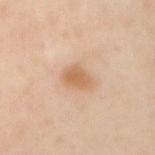Impression: Recorded during total-body skin imaging; not selected for excision or biopsy. Background: Imaged with cross-polarized lighting. Approximately 3 mm at its widest. Cropped from a whole-body photographic skin survey; the tile spans about 15 mm. The lesion is located on the left arm. A male patient aged 48 to 52.